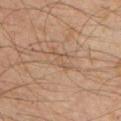<record>
<biopsy_status>not biopsied; imaged during a skin examination</biopsy_status>
<image>
  <source>total-body photography crop</source>
  <field_of_view_mm>15</field_of_view_mm>
</image>
<lesion_size>
  <long_diameter_mm_approx>3.0</long_diameter_mm_approx>
</lesion_size>
<site>front of the torso</site>
<patient>
  <sex>male</sex>
  <age_approx>30</age_approx>
</patient>
</record>